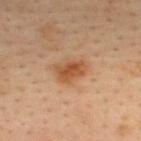site: upper back
patient:
  sex: male
  age_approx: 35
lighting: cross-polarized
automated_metrics:
  shape_asymmetry: 0.3
  lesion_detection_confidence_0_100: 100
image:
  source: total-body photography crop
  field_of_view_mm: 15